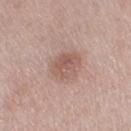workup = catalogued during a skin exam; not biopsied
anatomic site = the right thigh
diameter = ~3.5 mm (longest diameter)
image = ~15 mm crop, total-body skin-cancer survey
illumination = white-light illumination
subject = female, in their 60s